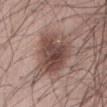Part of a total-body skin-imaging series; this lesion was reviewed on a skin check and was not flagged for biopsy.
From the abdomen.
Automated tile analysis of the lesion measured an area of roughly 20 mm² and an eccentricity of roughly 0.4. The software also gave roughly 13 lightness units darker than nearby skin.
Cropped from a total-body skin-imaging series; the visible field is about 15 mm.
A male patient, in their mid-40s.
Imaged with white-light lighting.